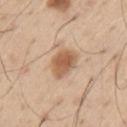Imaged during a routine full-body skin examination; the lesion was not biopsied and no histopathology is available.
A male subject in their mid-50s.
About 3.5 mm across.
This is a white-light tile.
From the arm.
Automated image analysis of the tile measured a footprint of about 8.5 mm², a shape eccentricity near 0.5, and a shape-asymmetry score of about 0.2 (0 = symmetric). The analysis additionally found a border-irregularity index near 2/10, internal color variation of about 3.5 on a 0–10 scale, and radial color variation of about 1. It also reported a nevus-likeness score of about 95/100.
A 15 mm close-up extracted from a 3D total-body photography capture.